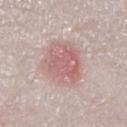Q: What are the patient's age and sex?
A: male, aged 63 to 67
Q: Lesion location?
A: the right lower leg
Q: What is the imaging modality?
A: 15 mm crop, total-body photography
Q: What lighting was used for the tile?
A: white-light illumination